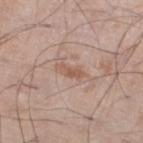Imaged during a routine full-body skin examination; the lesion was not biopsied and no histopathology is available. The lesion's longest dimension is about 3.5 mm. A 15 mm close-up extracted from a 3D total-body photography capture. A male subject, aged 68–72. Captured under white-light illumination. The lesion is located on the leg.